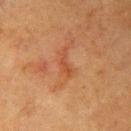Captured during whole-body skin photography for melanoma surveillance; the lesion was not biopsied.
Automated tile analysis of the lesion measured a lesion area of about 2.5 mm², an eccentricity of roughly 0.9, and two-axis asymmetry of about 0.75. The analysis additionally found a classifier nevus-likeness of about 0/100.
A close-up tile cropped from a whole-body skin photograph, about 15 mm across.
This is a cross-polarized tile.
The subject is a female roughly 55 years of age.
The lesion is on the right upper arm.